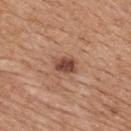Notes:
* workup — imaged on a skin check; not biopsied
* illumination — white-light illumination
* acquisition — ~15 mm crop, total-body skin-cancer survey
* anatomic site — the upper back
* automated metrics — a footprint of about 5 mm², an outline eccentricity of about 0.75 (0 = round, 1 = elongated), and two-axis asymmetry of about 0.25; about 13 CIELAB-L* units darker than the surrounding skin and a lesion-to-skin contrast of about 9.5 (normalized; higher = more distinct); a color-variation rating of about 3/10 and radial color variation of about 1
* size — ~3 mm (longest diameter)
* subject — female, in their mid- to late 40s